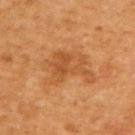Imaged during a routine full-body skin examination; the lesion was not biopsied and no histopathology is available.
Longest diameter approximately 6 mm.
A 15 mm crop from a total-body photograph taken for skin-cancer surveillance.
The tile uses cross-polarized illumination.
The lesion is located on the upper back.
Automated image analysis of the tile measured a lesion area of about 16 mm², an outline eccentricity of about 0.7 (0 = round, 1 = elongated), and a symmetry-axis asymmetry near 0.4. The software also gave a nevus-likeness score of about 5/100.
A male patient, aged 63–67.